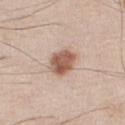biopsy status=imaged on a skin check; not biopsied | image-analysis metrics=border irregularity of about 1.5 on a 0–10 scale, a within-lesion color-variation index near 4.5/10, and radial color variation of about 1.5 | site=the chest | patient=male, roughly 45 years of age | imaging modality=15 mm crop, total-body photography.